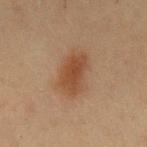{"biopsy_status": "not biopsied; imaged during a skin examination", "patient": {"sex": "male", "age_approx": 60}, "lighting": "cross-polarized", "lesion_size": {"long_diameter_mm_approx": 4.5}, "image": {"source": "total-body photography crop", "field_of_view_mm": 15}, "site": "left upper arm"}